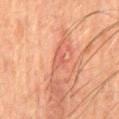Clinical impression:
Captured during whole-body skin photography for melanoma surveillance; the lesion was not biopsied.
Image and clinical context:
The patient is a male aged approximately 65. Located on the back. Cropped from a total-body skin-imaging series; the visible field is about 15 mm.